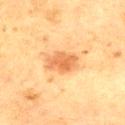Q: Is there a histopathology result?
A: total-body-photography surveillance lesion; no biopsy
Q: What kind of image is this?
A: ~15 mm tile from a whole-body skin photo
Q: Where on the body is the lesion?
A: the chest
Q: How large is the lesion?
A: ~3.5 mm (longest diameter)
Q: How was the tile lit?
A: cross-polarized illumination
Q: Patient demographics?
A: male, roughly 65 years of age